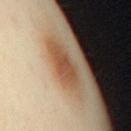<case>
  <biopsy_status>not biopsied; imaged during a skin examination</biopsy_status>
  <patient>
    <sex>female</sex>
    <age_approx>40</age_approx>
  </patient>
  <lesion_size>
    <long_diameter_mm_approx>4.0</long_diameter_mm_approx>
  </lesion_size>
  <site>mid back</site>
  <lighting>cross-polarized</lighting>
  <image>
    <source>total-body photography crop</source>
    <field_of_view_mm>15</field_of_view_mm>
  </image>
</case>